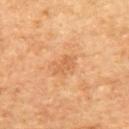– notes: catalogued during a skin exam; not biopsied
– acquisition: ~15 mm crop, total-body skin-cancer survey
– anatomic site: the upper back
– automated lesion analysis: about 7 CIELAB-L* units darker than the surrounding skin and a lesion-to-skin contrast of about 5 (normalized; higher = more distinct)
– subject: female, aged approximately 60
– lesion size: ≈2.5 mm
– illumination: cross-polarized